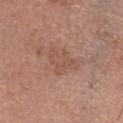Imaged during a routine full-body skin examination; the lesion was not biopsied and no histopathology is available.
On the head or neck.
Captured under white-light illumination.
A male subject, aged 58 to 62.
Approximately 3.5 mm at its widest.
Cropped from a total-body skin-imaging series; the visible field is about 15 mm.